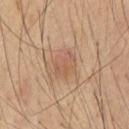Acquisition and patient details:
An algorithmic analysis of the crop reported a lesion color around L≈52 a*≈18 b*≈29 in CIELAB and a normalized border contrast of about 4.5. The software also gave a border-irregularity rating of about 2.5/10, internal color variation of about 3 on a 0–10 scale, and peripheral color asymmetry of about 1. Longest diameter approximately 3.5 mm. A lesion tile, about 15 mm wide, cut from a 3D total-body photograph. A male subject about 55 years old. From the abdomen.